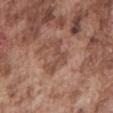Impression:
No biopsy was performed on this lesion — it was imaged during a full skin examination and was not determined to be concerning.
Background:
Longest diameter approximately 4.5 mm. A male subject about 75 years old. Cropped from a whole-body photographic skin survey; the tile spans about 15 mm. Captured under white-light illumination. Located on the mid back.